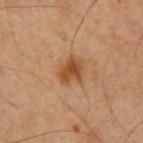{"biopsy_status": "not biopsied; imaged during a skin examination", "patient": {"sex": "male", "age_approx": 65}, "image": {"source": "total-body photography crop", "field_of_view_mm": 15}, "lighting": "cross-polarized", "site": "mid back", "automated_metrics": {"border_irregularity_0_10": 3.0, "color_variation_0_10": 4.0, "peripheral_color_asymmetry": 1.5}}